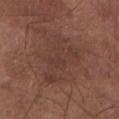Part of a total-body skin-imaging series; this lesion was reviewed on a skin check and was not flagged for biopsy.
A lesion tile, about 15 mm wide, cut from a 3D total-body photograph.
An algorithmic analysis of the crop reported a lesion area of about 16 mm² and a shape eccentricity near 0.8. And it measured an average lesion color of about L≈37 a*≈19 b*≈23 (CIELAB) and roughly 5 lightness units darker than nearby skin. And it measured an automated nevus-likeness rating near 0 out of 100.
A male subject aged 73 to 77.
The lesion is located on the right forearm.
The tile uses white-light illumination.